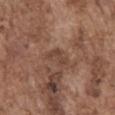Q: Was this lesion biopsied?
A: imaged on a skin check; not biopsied
Q: What are the patient's age and sex?
A: male, aged approximately 75
Q: How large is the lesion?
A: ≈3 mm
Q: What did automated image analysis measure?
A: an area of roughly 3.5 mm² and a shape-asymmetry score of about 0.6 (0 = symmetric)
Q: What is the imaging modality?
A: ~15 mm tile from a whole-body skin photo
Q: What is the anatomic site?
A: the abdomen